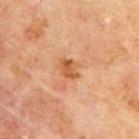Clinical impression: The lesion was tiled from a total-body skin photograph and was not biopsied. Background: A male patient, approximately 70 years of age. A region of skin cropped from a whole-body photographic capture, roughly 15 mm wide. From the upper back.